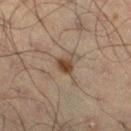Part of a total-body skin-imaging series; this lesion was reviewed on a skin check and was not flagged for biopsy. From the right thigh. A lesion tile, about 15 mm wide, cut from a 3D total-body photograph. About 2.5 mm across. Captured under cross-polarized illumination. A male subject aged 48–52.